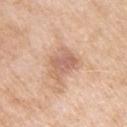Assessment:
This lesion was catalogued during total-body skin photography and was not selected for biopsy.
Background:
Cropped from a total-body skin-imaging series; the visible field is about 15 mm. A female subject, aged around 75. Located on the left upper arm. About 3.5 mm across. The tile uses white-light illumination.